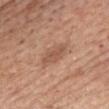{
  "biopsy_status": "not biopsied; imaged during a skin examination",
  "patient": {
    "sex": "male",
    "age_approx": 60
  },
  "image": {
    "source": "total-body photography crop",
    "field_of_view_mm": 15
  },
  "lighting": "white-light",
  "site": "head or neck"
}